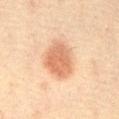patient = female, roughly 65 years of age
location = the front of the torso
acquisition = ~15 mm tile from a whole-body skin photo